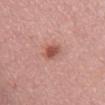Clinical impression: No biopsy was performed on this lesion — it was imaged during a full skin examination and was not determined to be concerning. Acquisition and patient details: Imaged with white-light lighting. A female subject roughly 70 years of age. An algorithmic analysis of the crop reported a mean CIELAB color near L≈53 a*≈26 b*≈28, about 11 CIELAB-L* units darker than the surrounding skin, and a normalized lesion–skin contrast near 8. It also reported border irregularity of about 2 on a 0–10 scale, internal color variation of about 3.5 on a 0–10 scale, and radial color variation of about 1. And it measured a detector confidence of about 100 out of 100 that the crop contains a lesion. The lesion's longest dimension is about 2.5 mm. From the front of the torso. Cropped from a total-body skin-imaging series; the visible field is about 15 mm.